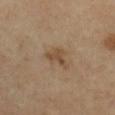Recorded during total-body skin imaging; not selected for excision or biopsy. A roughly 15 mm field-of-view crop from a total-body skin photograph. Automated tile analysis of the lesion measured an average lesion color of about L≈48 a*≈15 b*≈31 (CIELAB), roughly 8 lightness units darker than nearby skin, and a normalized border contrast of about 6.5. And it measured a border-irregularity rating of about 5/10 and peripheral color asymmetry of about 1. It also reported a classifier nevus-likeness of about 10/100 and lesion-presence confidence of about 100/100. About 3.5 mm across. The subject is a male aged approximately 65. The lesion is on the right lower leg. The tile uses cross-polarized illumination.